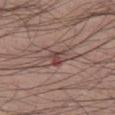The lesion was tiled from a total-body skin photograph and was not biopsied. A lesion tile, about 15 mm wide, cut from a 3D total-body photograph. The subject is a male in their 40s. Located on the left thigh. About 3 mm across. This is a white-light tile.